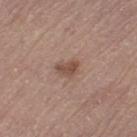No biopsy was performed on this lesion — it was imaged during a full skin examination and was not determined to be concerning. The recorded lesion diameter is about 3 mm. This is a white-light tile. A female patient aged 63–67. The lesion is on the left thigh. Cropped from a total-body skin-imaging series; the visible field is about 15 mm.